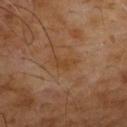Located on the upper back. A 15 mm close-up extracted from a 3D total-body photography capture. A male subject aged 53 to 57.